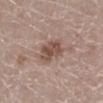Case summary:
- workup · total-body-photography surveillance lesion; no biopsy
- illumination · white-light illumination
- subject · female, aged approximately 50
- anatomic site · the right lower leg
- image source · total-body-photography crop, ~15 mm field of view